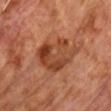Q: Was a biopsy performed?
A: total-body-photography surveillance lesion; no biopsy
Q: Who is the patient?
A: male, aged 68 to 72
Q: How was this image acquired?
A: total-body-photography crop, ~15 mm field of view
Q: What is the lesion's diameter?
A: ~5.5 mm (longest diameter)
Q: Where on the body is the lesion?
A: the front of the torso
Q: How was the tile lit?
A: cross-polarized illumination